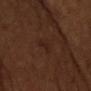The total-body-photography lesion software estimated a footprint of about 2.5 mm² and an eccentricity of roughly 0.9. It also reported peripheral color asymmetry of about 0. The analysis additionally found a classifier nevus-likeness of about 0/100. Captured under cross-polarized illumination. On the chest. A male subject in their 60s. A close-up tile cropped from a whole-body skin photograph, about 15 mm across.